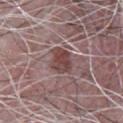automated metrics: an average lesion color of about L≈43 a*≈20 b*≈19 (CIELAB), about 11 CIELAB-L* units darker than the surrounding skin, and a normalized lesion–skin contrast near 9; an automated nevus-likeness rating near 95 out of 100 and a detector confidence of about 85 out of 100 that the crop contains a lesion | subject: male, approximately 65 years of age | lesion diameter: ≈3.5 mm | location: the chest | image: total-body-photography crop, ~15 mm field of view | lighting: white-light illumination.